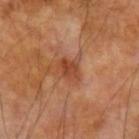illumination: cross-polarized | site: the left forearm | lesion diameter: about 3 mm | image source: 15 mm crop, total-body photography | subject: male, aged around 70.